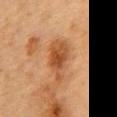Findings:
• biopsy status · total-body-photography surveillance lesion; no biopsy
• lighting · cross-polarized illumination
• patient · male, aged 83 or over
• image · total-body-photography crop, ~15 mm field of view
• automated metrics · a lesion area of about 10 mm²; an average lesion color of about L≈44 a*≈23 b*≈35 (CIELAB) and about 10 CIELAB-L* units darker than the surrounding skin; a border-irregularity index near 4.5/10, a within-lesion color-variation index near 4/10, and radial color variation of about 1
• size · ≈5 mm
• anatomic site · the chest